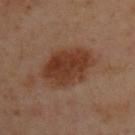The lesion was tiled from a total-body skin photograph and was not biopsied.
A 15 mm crop from a total-body photograph taken for skin-cancer surveillance.
On the upper back.
A male subject roughly 50 years of age.